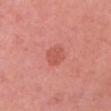| feature | finding |
|---|---|
| follow-up | total-body-photography surveillance lesion; no biopsy |
| anatomic site | the head or neck |
| lighting | white-light illumination |
| imaging modality | 15 mm crop, total-body photography |
| patient | female, roughly 65 years of age |